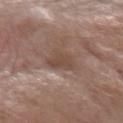This lesion was catalogued during total-body skin photography and was not selected for biopsy. Approximately 3 mm at its widest. A male subject, aged 68–72. On the arm. This is a white-light tile. A close-up tile cropped from a whole-body skin photograph, about 15 mm across.